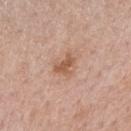Imaged during a routine full-body skin examination; the lesion was not biopsied and no histopathology is available. Located on the arm. This is a white-light tile. The recorded lesion diameter is about 3 mm. The total-body-photography lesion software estimated a lesion area of about 4.5 mm². It also reported a mean CIELAB color near L≈56 a*≈21 b*≈31, about 10 CIELAB-L* units darker than the surrounding skin, and a normalized lesion–skin contrast near 7.5. The analysis additionally found a border-irregularity index near 4.5/10, internal color variation of about 1 on a 0–10 scale, and radial color variation of about 0.5. The software also gave a nevus-likeness score of about 35/100. A female patient, aged approximately 40. This image is a 15 mm lesion crop taken from a total-body photograph.